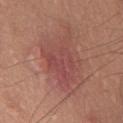| key | value |
|---|---|
| follow-up | catalogued during a skin exam; not biopsied |
| body site | the chest |
| acquisition | total-body-photography crop, ~15 mm field of view |
| patient | male, aged 53 to 57 |
| TBP lesion metrics | a lesion color around L≈47 a*≈26 b*≈24 in CIELAB, about 7 CIELAB-L* units darker than the surrounding skin, and a lesion-to-skin contrast of about 6 (normalized; higher = more distinct); radial color variation of about 1; a classifier nevus-likeness of about 5/100 and lesion-presence confidence of about 100/100 |
| lesion size | ≈6.5 mm |